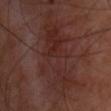Assessment: Imaged during a routine full-body skin examination; the lesion was not biopsied and no histopathology is available. Clinical summary: The tile uses cross-polarized illumination. The patient is a male aged 48–52. A 15 mm close-up tile from a total-body photography series done for melanoma screening. Longest diameter approximately 10 mm. From the front of the torso.